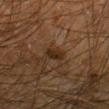Acquisition and patient details: The subject is a male about 65 years old. This image is a 15 mm lesion crop taken from a total-body photograph. This is a cross-polarized tile. From the left forearm. Approximately 2.5 mm at its widest.